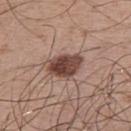No biopsy was performed on this lesion — it was imaged during a full skin examination and was not determined to be concerning.
This image is a 15 mm lesion crop taken from a total-body photograph.
A male patient, aged approximately 50.
Captured under white-light illumination.
Approximately 4 mm at its widest.
Located on the upper back.